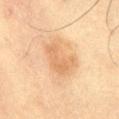Q: Was this lesion biopsied?
A: catalogued during a skin exam; not biopsied
Q: Where on the body is the lesion?
A: the abdomen
Q: What is the lesion's diameter?
A: about 4.5 mm
Q: What did automated image analysis measure?
A: an average lesion color of about L≈57 a*≈18 b*≈34 (CIELAB); a border-irregularity index near 4/10 and internal color variation of about 2 on a 0–10 scale; a detector confidence of about 100 out of 100 that the crop contains a lesion
Q: How was this image acquired?
A: total-body-photography crop, ~15 mm field of view
Q: Who is the patient?
A: male, approximately 60 years of age